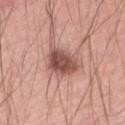Background:
A 15 mm close-up extracted from a 3D total-body photography capture. Longest diameter approximately 4 mm. Captured under white-light illumination. Located on the back. A male subject, aged around 60.